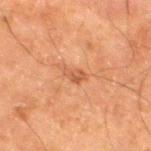notes: no biopsy performed (imaged during a skin exam)
anatomic site: the right thigh
subject: male, aged around 80
tile lighting: cross-polarized illumination
size: ≈3 mm
acquisition: ~15 mm crop, total-body skin-cancer survey